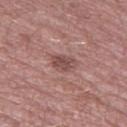follow-up = no biopsy performed (imaged during a skin exam) | subject = male, aged approximately 55 | diameter = about 3 mm | imaging modality = ~15 mm tile from a whole-body skin photo | illumination = white-light | location = the left thigh.